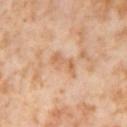Q: What is the imaging modality?
A: ~15 mm crop, total-body skin-cancer survey
Q: Lesion size?
A: ≈3.5 mm
Q: Patient demographics?
A: female, about 55 years old
Q: Where on the body is the lesion?
A: the right thigh
Q: What lighting was used for the tile?
A: cross-polarized illumination